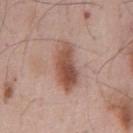This lesion was catalogued during total-body skin photography and was not selected for biopsy. A region of skin cropped from a whole-body photographic capture, roughly 15 mm wide. A male patient aged 53 to 57. The lesion is on the chest. The recorded lesion diameter is about 6 mm. The tile uses white-light illumination.